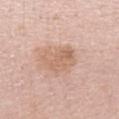A lesion tile, about 15 mm wide, cut from a 3D total-body photograph.
Approximately 4 mm at its widest.
On the left arm.
A female patient roughly 40 years of age.
Captured under white-light illumination.